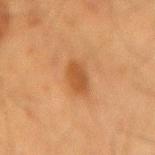Part of a total-body skin-imaging series; this lesion was reviewed on a skin check and was not flagged for biopsy. A male subject in their mid- to late 60s. On the lower back. Automated tile analysis of the lesion measured about 8 CIELAB-L* units darker than the surrounding skin and a lesion-to-skin contrast of about 7 (normalized; higher = more distinct). Cropped from a whole-body photographic skin survey; the tile spans about 15 mm. The tile uses cross-polarized illumination. The lesion's longest dimension is about 3 mm.